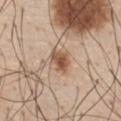Clinical impression: Recorded during total-body skin imaging; not selected for excision or biopsy. Clinical summary: Approximately 3 mm at its widest. Cropped from a whole-body photographic skin survey; the tile spans about 15 mm. Captured under white-light illumination. A male patient aged approximately 60. The lesion-visualizer software estimated an area of roughly 6 mm², a shape eccentricity near 0.65, and a shape-asymmetry score of about 0.3 (0 = symmetric). The analysis additionally found a lesion color around L≈55 a*≈18 b*≈32 in CIELAB, a lesion–skin lightness drop of about 13, and a normalized border contrast of about 9. The software also gave a border-irregularity rating of about 3/10, a within-lesion color-variation index near 4.5/10, and radial color variation of about 1. And it measured a nevus-likeness score of about 95/100 and a lesion-detection confidence of about 100/100. The lesion is on the front of the torso.